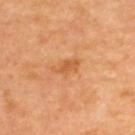  biopsy_status: not biopsied; imaged during a skin examination
  image:
    source: total-body photography crop
    field_of_view_mm: 15
  lesion_size:
    long_diameter_mm_approx: 3.5
  lighting: cross-polarized
  patient:
    age_approx: 65
  site: upper back